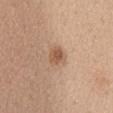<lesion>
  <biopsy_status>not biopsied; imaged during a skin examination</biopsy_status>
  <patient>
    <sex>female</sex>
    <age_approx>45</age_approx>
  </patient>
  <site>chest</site>
  <lighting>white-light</lighting>
  <image>
    <source>total-body photography crop</source>
    <field_of_view_mm>15</field_of_view_mm>
  </image>
  <lesion_size>
    <long_diameter_mm_approx>2.5</long_diameter_mm_approx>
  </lesion_size>
</lesion>